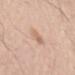Imaged during a routine full-body skin examination; the lesion was not biopsied and no histopathology is available. The lesion-visualizer software estimated a lesion color around L≈66 a*≈19 b*≈30 in CIELAB and a normalized border contrast of about 5. The software also gave border irregularity of about 2.5 on a 0–10 scale and radial color variation of about 1. The software also gave lesion-presence confidence of about 100/100. The patient is a male aged approximately 65. A 15 mm close-up extracted from a 3D total-body photography capture. From the left upper arm. Measured at roughly 3 mm in maximum diameter.